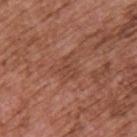Impression: Part of a total-body skin-imaging series; this lesion was reviewed on a skin check and was not flagged for biopsy. Clinical summary: Cropped from a total-body skin-imaging series; the visible field is about 15 mm. A male patient, aged around 75. Captured under white-light illumination. The lesion is located on the upper back. The lesion-visualizer software estimated a lesion-detection confidence of about 95/100. Approximately 3 mm at its widest.